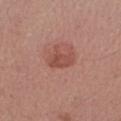Recorded during total-body skin imaging; not selected for excision or biopsy. A male subject aged 43 to 47. Longest diameter approximately 2.5 mm. The lesion-visualizer software estimated roughly 8 lightness units darker than nearby skin and a lesion-to-skin contrast of about 6.5 (normalized; higher = more distinct). The analysis additionally found a classifier nevus-likeness of about 90/100 and a detector confidence of about 100 out of 100 that the crop contains a lesion. A 15 mm close-up tile from a total-body photography series done for melanoma screening. Imaged with white-light lighting. The lesion is on the right forearm.